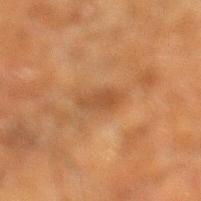Q: Who is the patient?
A: male, in their 60s
Q: What is the imaging modality?
A: total-body-photography crop, ~15 mm field of view
Q: Where on the body is the lesion?
A: the left lower leg